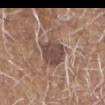The lesion was tiled from a total-body skin photograph and was not biopsied.
A 15 mm crop from a total-body photograph taken for skin-cancer surveillance.
A male subject, in their 80s.
The tile uses white-light illumination.
On the head or neck.
The lesion's longest dimension is about 4 mm.